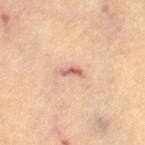A roughly 15 mm field-of-view crop from a total-body skin photograph. From the leg. A female patient aged 63–67.